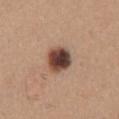No biopsy was performed on this lesion — it was imaged during a full skin examination and was not determined to be concerning. Located on the back. The lesion's longest dimension is about 3.5 mm. A close-up tile cropped from a whole-body skin photograph, about 15 mm across. Imaged with white-light lighting. A female subject, about 30 years old.